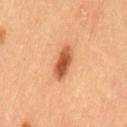Assessment: No biopsy was performed on this lesion — it was imaged during a full skin examination and was not determined to be concerning. Acquisition and patient details: About 4 mm across. A female patient, in their 60s. A 15 mm close-up tile from a total-body photography series done for melanoma screening. The tile uses cross-polarized illumination. The lesion is on the lower back.